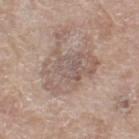The total-body-photography lesion software estimated a lesion color around L≈56 a*≈14 b*≈22 in CIELAB, a lesion–skin lightness drop of about 8, and a lesion-to-skin contrast of about 5.5 (normalized; higher = more distinct). The analysis additionally found a nevus-likeness score of about 0/100 and lesion-presence confidence of about 80/100. This is a white-light tile. From the right thigh. A lesion tile, about 15 mm wide, cut from a 3D total-body photograph. Longest diameter approximately 6.5 mm. A female subject, aged 73–77.